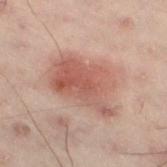Recorded during total-body skin imaging; not selected for excision or biopsy. Imaged with cross-polarized lighting. From the left lower leg. A male subject aged around 50. Measured at roughly 7.5 mm in maximum diameter. This image is a 15 mm lesion crop taken from a total-body photograph.